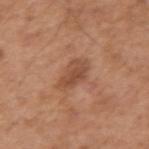This lesion was catalogued during total-body skin photography and was not selected for biopsy. An algorithmic analysis of the crop reported a mean CIELAB color near L≈49 a*≈23 b*≈32, roughly 9 lightness units darker than nearby skin, and a normalized lesion–skin contrast near 7. Located on the right upper arm. A male subject, aged 53 to 57. Approximately 4 mm at its widest. This is a white-light tile. A 15 mm crop from a total-body photograph taken for skin-cancer surveillance.